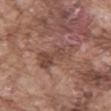Findings:
• notes — imaged on a skin check; not biopsied
• patient — male, approximately 75 years of age
• anatomic site — the front of the torso
• acquisition — total-body-photography crop, ~15 mm field of view
• illumination — white-light illumination
• diameter — ~6 mm (longest diameter)